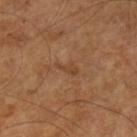<lesion>
  <biopsy_status>not biopsied; imaged during a skin examination</biopsy_status>
</lesion>